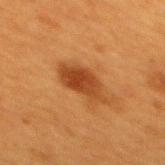Located on the upper back.
The tile uses cross-polarized illumination.
A female patient, aged around 40.
A 15 mm close-up tile from a total-body photography series done for melanoma screening.